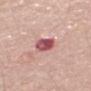Case summary:
* anatomic site — the mid back
* lighting — white-light illumination
* TBP lesion metrics — a border-irregularity rating of about 1.5/10 and peripheral color asymmetry of about 2; a detector confidence of about 100 out of 100 that the crop contains a lesion
* lesion size — ~3 mm (longest diameter)
* subject — male, approximately 70 years of age
* image source — 15 mm crop, total-body photography